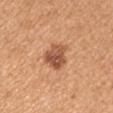body site — the arm; image source — ~15 mm crop, total-body skin-cancer survey; illumination — white-light; subject — female, in their mid-40s.